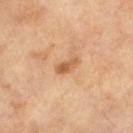This lesion was catalogued during total-body skin photography and was not selected for biopsy. Approximately 3 mm at its widest. Imaged with cross-polarized lighting. The lesion-visualizer software estimated a mean CIELAB color near L≈57 a*≈22 b*≈39, about 11 CIELAB-L* units darker than the surrounding skin, and a normalized border contrast of about 7.5. The analysis additionally found a classifier nevus-likeness of about 30/100. A roughly 15 mm field-of-view crop from a total-body skin photograph. A male patient, aged 63–67.